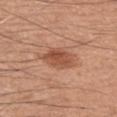Q: Where on the body is the lesion?
A: the chest
Q: How was this image acquired?
A: ~15 mm tile from a whole-body skin photo
Q: What are the patient's age and sex?
A: male, in their 60s